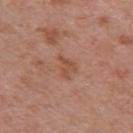Q: Was a biopsy performed?
A: no biopsy performed (imaged during a skin exam)